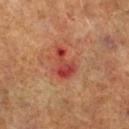follow-up: imaged on a skin check; not biopsied
acquisition: ~15 mm tile from a whole-body skin photo
anatomic site: the leg
TBP lesion metrics: a lesion area of about 6.5 mm² and a shape eccentricity near 0.8; a mean CIELAB color near L≈36 a*≈29 b*≈26, about 9 CIELAB-L* units darker than the surrounding skin, and a normalized border contrast of about 8; an automated nevus-likeness rating near 0 out of 100 and a lesion-detection confidence of about 100/100
patient: male, about 70 years old
diameter: about 4 mm
illumination: cross-polarized illumination